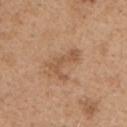Captured during whole-body skin photography for melanoma surveillance; the lesion was not biopsied. A male subject, in their mid- to late 60s. The lesion-visualizer software estimated a border-irregularity index near 8/10, internal color variation of about 0.5 on a 0–10 scale, and a peripheral color-asymmetry measure near 0. And it measured a nevus-likeness score of about 0/100 and lesion-presence confidence of about 100/100. A close-up tile cropped from a whole-body skin photograph, about 15 mm across. The recorded lesion diameter is about 4 mm. The lesion is on the chest. This is a white-light tile.